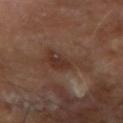Q: Is there a histopathology result?
A: no biopsy performed (imaged during a skin exam)
Q: How was the tile lit?
A: cross-polarized
Q: What are the patient's age and sex?
A: male, approximately 65 years of age
Q: How large is the lesion?
A: ≈5 mm
Q: How was this image acquired?
A: total-body-photography crop, ~15 mm field of view
Q: Lesion location?
A: the right forearm